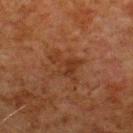The lesion was tiled from a total-body skin photograph and was not biopsied.
About 4 mm across.
The lesion is on the upper back.
Automated tile analysis of the lesion measured a symmetry-axis asymmetry near 0.5. The software also gave about 5 CIELAB-L* units darker than the surrounding skin and a normalized lesion–skin contrast near 5.5. And it measured border irregularity of about 6.5 on a 0–10 scale, a color-variation rating of about 2.5/10, and radial color variation of about 1.
The patient is a male approximately 80 years of age.
This is a cross-polarized tile.
A close-up tile cropped from a whole-body skin photograph, about 15 mm across.